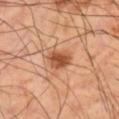workup: no biopsy performed (imaged during a skin exam); illumination: cross-polarized illumination; patient: male, aged approximately 50; site: the leg; size: about 3 mm; imaging modality: ~15 mm crop, total-body skin-cancer survey.